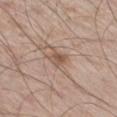Findings:
- biopsy status · imaged on a skin check; not biopsied
- tile lighting · white-light
- imaging modality · ~15 mm crop, total-body skin-cancer survey
- anatomic site · the right thigh
- automated lesion analysis · a footprint of about 3.5 mm², a shape eccentricity near 0.7, and a shape-asymmetry score of about 0.3 (0 = symmetric); a border-irregularity rating of about 2.5/10
- subject · male, approximately 60 years of age